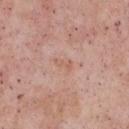{
  "biopsy_status": "not biopsied; imaged during a skin examination",
  "patient": {
    "sex": "male",
    "age_approx": 55
  },
  "lesion_size": {
    "long_diameter_mm_approx": 2.5
  },
  "site": "chest",
  "image": {
    "source": "total-body photography crop",
    "field_of_view_mm": 15
  },
  "lighting": "white-light"
}